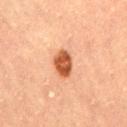Assessment:
Imaged during a routine full-body skin examination; the lesion was not biopsied and no histopathology is available.
Acquisition and patient details:
The subject is a female roughly 70 years of age. From the lower back. Cropped from a total-body skin-imaging series; the visible field is about 15 mm.